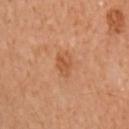Impression:
The lesion was photographed on a routine skin check and not biopsied; there is no pathology result.
Context:
Cropped from a whole-body photographic skin survey; the tile spans about 15 mm. The subject is a female roughly 60 years of age. Captured under cross-polarized illumination. The total-body-photography lesion software estimated an area of roughly 5 mm² and a shape eccentricity near 0.7. The analysis additionally found a lesion color around L≈47 a*≈22 b*≈33 in CIELAB and a normalized lesion–skin contrast near 6. The analysis additionally found a border-irregularity rating of about 2.5/10, internal color variation of about 2.5 on a 0–10 scale, and a peripheral color-asymmetry measure near 1. On the right arm. The lesion's longest dimension is about 3 mm.